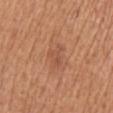Clinical summary:
A region of skin cropped from a whole-body photographic capture, roughly 15 mm wide. Imaged with white-light lighting. The subject is a female about 55 years old. On the right upper arm. The lesion's longest dimension is about 3 mm. The lesion-visualizer software estimated an outline eccentricity of about 0.7 (0 = round, 1 = elongated). It also reported border irregularity of about 3.5 on a 0–10 scale, a within-lesion color-variation index near 2/10, and a peripheral color-asymmetry measure near 1. It also reported a detector confidence of about 100 out of 100 that the crop contains a lesion.